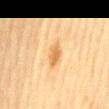workup = catalogued during a skin exam; not biopsied
image = ~15 mm tile from a whole-body skin photo
lesion diameter = ~3.5 mm (longest diameter)
anatomic site = the mid back
patient = female, aged around 55
illumination = cross-polarized